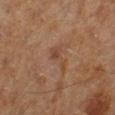Assessment: The lesion was photographed on a routine skin check and not biopsied; there is no pathology result. Image and clinical context: The lesion is on the left lower leg. The patient is a male about 60 years old. This image is a 15 mm lesion crop taken from a total-body photograph. The total-body-photography lesion software estimated an average lesion color of about L≈41 a*≈19 b*≈28 (CIELAB), about 6 CIELAB-L* units darker than the surrounding skin, and a normalized lesion–skin contrast near 5.5. The software also gave a classifier nevus-likeness of about 0/100.